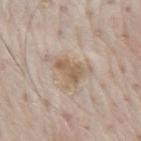Clinical impression: The lesion was photographed on a routine skin check and not biopsied; there is no pathology result. Clinical summary: On the mid back. Measured at roughly 5 mm in maximum diameter. This is a white-light tile. Automated image analysis of the tile measured a lesion area of about 9.5 mm² and an eccentricity of roughly 0.7. The software also gave a border-irregularity rating of about 6.5/10, a color-variation rating of about 3.5/10, and peripheral color asymmetry of about 1.5. The software also gave a lesion-detection confidence of about 90/100. A male subject, roughly 80 years of age. Cropped from a whole-body photographic skin survey; the tile spans about 15 mm.